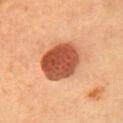| field | value |
|---|---|
| biopsy status | imaged on a skin check; not biopsied |
| patient | female |
| site | the chest |
| size | about 5.5 mm |
| lighting | cross-polarized illumination |
| acquisition | ~15 mm crop, total-body skin-cancer survey |